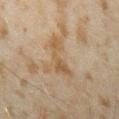Image and clinical context:
The lesion's longest dimension is about 4.5 mm. Captured under cross-polarized illumination. Automated tile analysis of the lesion measured a symmetry-axis asymmetry near 0.6. A 15 mm close-up tile from a total-body photography series done for melanoma screening. The subject is a male in their mid-40s. On the right forearm.